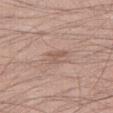Impression:
Part of a total-body skin-imaging series; this lesion was reviewed on a skin check and was not flagged for biopsy.
Image and clinical context:
Captured under white-light illumination. A male patient, approximately 35 years of age. Measured at roughly 2.5 mm in maximum diameter. The lesion is on the right thigh. A region of skin cropped from a whole-body photographic capture, roughly 15 mm wide.